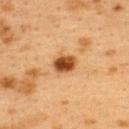follow-up = no biopsy performed (imaged during a skin exam); anatomic site = the upper back; imaging modality = 15 mm crop, total-body photography; subject = female, in their 40s; tile lighting = cross-polarized illumination.